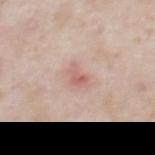biopsy status = total-body-photography surveillance lesion; no biopsy
patient = male, approximately 60 years of age
diameter = ≈2.5 mm
imaging modality = 15 mm crop, total-body photography
location = the abdomen